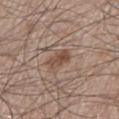Assessment:
The lesion was tiled from a total-body skin photograph and was not biopsied.
Context:
The total-body-photography lesion software estimated a lesion area of about 5 mm², an eccentricity of roughly 0.85, and two-axis asymmetry of about 0.25. The software also gave a mean CIELAB color near L≈48 a*≈18 b*≈26, about 9 CIELAB-L* units darker than the surrounding skin, and a normalized lesion–skin contrast near 7.5. It also reported a classifier nevus-likeness of about 80/100 and lesion-presence confidence of about 100/100. The lesion is located on the left lower leg. Measured at roughly 3.5 mm in maximum diameter. Imaged with white-light lighting. A male patient in their 60s. A region of skin cropped from a whole-body photographic capture, roughly 15 mm wide.